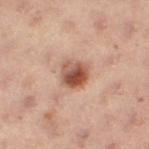| field | value |
|---|---|
| workup | total-body-photography surveillance lesion; no biopsy |
| location | the left leg |
| image source | ~15 mm crop, total-body skin-cancer survey |
| lesion diameter | about 3.5 mm |
| illumination | cross-polarized |
| subject | female, approximately 55 years of age |
| automated metrics | an area of roughly 8 mm², an outline eccentricity of about 0.55 (0 = round, 1 = elongated), and a shape-asymmetry score of about 0.2 (0 = symmetric); a lesion color around L≈44 a*≈20 b*≈25 in CIELAB, roughly 13 lightness units darker than nearby skin, and a normalized lesion–skin contrast near 10; an automated nevus-likeness rating near 95 out of 100 and lesion-presence confidence of about 100/100 |